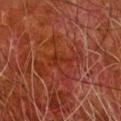This lesion was catalogued during total-body skin photography and was not selected for biopsy.